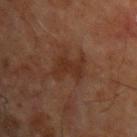Part of a total-body skin-imaging series; this lesion was reviewed on a skin check and was not flagged for biopsy. Cropped from a whole-body photographic skin survey; the tile spans about 15 mm. A male subject, aged around 70. The lesion is located on the chest.